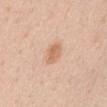Q: Was this lesion biopsied?
A: total-body-photography surveillance lesion; no biopsy
Q: How was this image acquired?
A: ~15 mm tile from a whole-body skin photo
Q: Who is the patient?
A: male, aged around 35
Q: What did automated image analysis measure?
A: a footprint of about 4.5 mm², a shape eccentricity near 0.7, and a shape-asymmetry score of about 0.25 (0 = symmetric); border irregularity of about 2.5 on a 0–10 scale, internal color variation of about 2 on a 0–10 scale, and peripheral color asymmetry of about 0.5; a classifier nevus-likeness of about 85/100 and lesion-presence confidence of about 100/100
Q: What is the anatomic site?
A: the mid back
Q: Illumination type?
A: white-light
Q: What is the lesion's diameter?
A: ≈2.5 mm